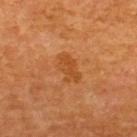Clinical impression: Part of a total-body skin-imaging series; this lesion was reviewed on a skin check and was not flagged for biopsy. Clinical summary: The total-body-photography lesion software estimated a lesion color around L≈45 a*≈26 b*≈41 in CIELAB, about 7 CIELAB-L* units darker than the surrounding skin, and a normalized lesion–skin contrast near 6. It also reported a within-lesion color-variation index near 2/10 and peripheral color asymmetry of about 1. From the upper back. About 4 mm across. Imaged with cross-polarized lighting. The subject is a male in their 60s. A lesion tile, about 15 mm wide, cut from a 3D total-body photograph.